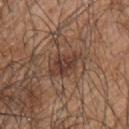Recorded during total-body skin imaging; not selected for excision or biopsy. A lesion tile, about 15 mm wide, cut from a 3D total-body photograph. The lesion is located on the upper back. A male patient, aged approximately 45. Imaged with white-light lighting.